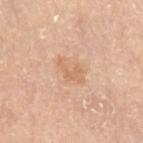Notes:
* notes — imaged on a skin check; not biopsied
* automated lesion analysis — border irregularity of about 6 on a 0–10 scale, a color-variation rating of about 1/10, and a peripheral color-asymmetry measure near 0.5
* imaging modality — ~15 mm tile from a whole-body skin photo
* diameter — about 3 mm
* patient — male, aged 63 to 67
* location — the left upper arm
* illumination — white-light